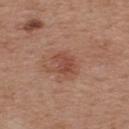Assessment: No biopsy was performed on this lesion — it was imaged during a full skin examination and was not determined to be concerning. Context: A region of skin cropped from a whole-body photographic capture, roughly 15 mm wide. From the upper back. Captured under white-light illumination. A male subject in their mid- to late 50s. About 3 mm across.